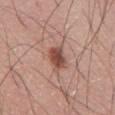Part of a total-body skin-imaging series; this lesion was reviewed on a skin check and was not flagged for biopsy. Cropped from a total-body skin-imaging series; the visible field is about 15 mm. The lesion is on the abdomen. This is a white-light tile. Automated tile analysis of the lesion measured a lesion area of about 5.5 mm² and an outline eccentricity of about 0.7 (0 = round, 1 = elongated). The software also gave an automated nevus-likeness rating near 90 out of 100 and a lesion-detection confidence of about 100/100. Longest diameter approximately 3 mm. The subject is a male in their 50s.